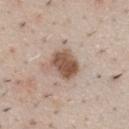Clinical impression: Part of a total-body skin-imaging series; this lesion was reviewed on a skin check and was not flagged for biopsy. Context: Located on the chest. The tile uses white-light illumination. The total-body-photography lesion software estimated an area of roughly 10 mm², an outline eccentricity of about 0.55 (0 = round, 1 = elongated), and two-axis asymmetry of about 0.2. It also reported about 14 CIELAB-L* units darker than the surrounding skin and a lesion-to-skin contrast of about 10 (normalized; higher = more distinct). It also reported a border-irregularity rating of about 2/10, a color-variation rating of about 4/10, and a peripheral color-asymmetry measure near 1.5. The analysis additionally found an automated nevus-likeness rating near 90 out of 100 and lesion-presence confidence of about 100/100. A male patient aged 48 to 52. This image is a 15 mm lesion crop taken from a total-body photograph. Approximately 4 mm at its widest.